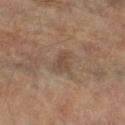This lesion was catalogued during total-body skin photography and was not selected for biopsy. Automated image analysis of the tile measured a mean CIELAB color near L≈45 a*≈15 b*≈27, a lesion–skin lightness drop of about 7, and a lesion-to-skin contrast of about 5.5 (normalized; higher = more distinct). The software also gave an automated nevus-likeness rating near 0 out of 100 and a lesion-detection confidence of about 100/100. The lesion's longest dimension is about 2.5 mm. A male subject in their 70s. From the left forearm. Imaged with cross-polarized lighting. A 15 mm crop from a total-body photograph taken for skin-cancer surveillance.